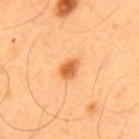Context: The subject is a male roughly 55 years of age. The lesion is on the back. A roughly 15 mm field-of-view crop from a total-body skin photograph. This is a cross-polarized tile.